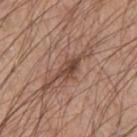biopsy_status: not biopsied; imaged during a skin examination
lighting: white-light
automated_metrics:
  area_mm2_approx: 5.0
  eccentricity: 0.85
  shape_asymmetry: 0.3
  cielab_L: 45
  cielab_a: 19
  cielab_b: 26
  vs_skin_darker_L: 11.0
  vs_skin_contrast_norm: 8.0
  nevus_likeness_0_100: 50
  lesion_detection_confidence_0_100: 95
lesion_size:
  long_diameter_mm_approx: 3.5
site: right upper arm
image:
  source: total-body photography crop
  field_of_view_mm: 15
patient:
  sex: male
  age_approx: 50